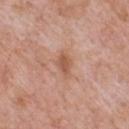Clinical impression:
Captured during whole-body skin photography for melanoma surveillance; the lesion was not biopsied.
Image and clinical context:
The recorded lesion diameter is about 3 mm. A lesion tile, about 15 mm wide, cut from a 3D total-body photograph. Automated tile analysis of the lesion measured an area of roughly 4 mm², an outline eccentricity of about 0.8 (0 = round, 1 = elongated), and a symmetry-axis asymmetry near 0.3. And it measured a lesion–skin lightness drop of about 9 and a normalized border contrast of about 7. The analysis additionally found an automated nevus-likeness rating near 0 out of 100 and a detector confidence of about 100 out of 100 that the crop contains a lesion. Captured under white-light illumination. The patient is a male aged approximately 60. The lesion is located on the chest.